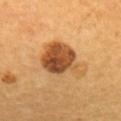| field | value |
|---|---|
| notes | catalogued during a skin exam; not biopsied |
| automated metrics | a lesion area of about 17 mm² and two-axis asymmetry of about 0.3; an average lesion color of about L≈50 a*≈23 b*≈40 (CIELAB) and a normalized border contrast of about 11; an automated nevus-likeness rating near 85 out of 100 and a lesion-detection confidence of about 100/100 |
| body site | the upper back |
| tile lighting | cross-polarized illumination |
| image | ~15 mm crop, total-body skin-cancer survey |
| diameter | ~6 mm (longest diameter) |
| subject | female, aged 48 to 52 |